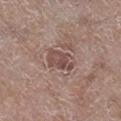Clinical impression: The lesion was tiled from a total-body skin photograph and was not biopsied. Clinical summary: Captured under white-light illumination. A male subject about 80 years old. A roughly 15 mm field-of-view crop from a total-body skin photograph. The recorded lesion diameter is about 4 mm. From the right lower leg.